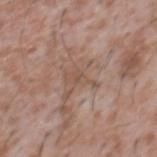Case summary:
* follow-up · catalogued during a skin exam; not biopsied
* diameter · ≈3.5 mm
* image · total-body-photography crop, ~15 mm field of view
* tile lighting · white-light illumination
* patient · male, aged 43–47
* site · the chest
* TBP lesion metrics · a mean CIELAB color near L≈52 a*≈18 b*≈27, a lesion–skin lightness drop of about 7, and a lesion-to-skin contrast of about 5 (normalized; higher = more distinct); a color-variation rating of about 1/10 and a peripheral color-asymmetry measure near 0.5; an automated nevus-likeness rating near 0 out of 100 and a lesion-detection confidence of about 80/100